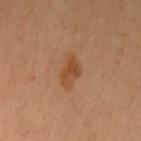workup: imaged on a skin check; not biopsied | lighting: cross-polarized | automated metrics: a lesion color around L≈48 a*≈22 b*≈37 in CIELAB, about 8 CIELAB-L* units darker than the surrounding skin, and a normalized lesion–skin contrast near 7.5; a border-irregularity index near 4/10, internal color variation of about 2.5 on a 0–10 scale, and radial color variation of about 1 | subject: female, in their 40s | imaging modality: 15 mm crop, total-body photography | size: ~3.5 mm (longest diameter) | location: the left upper arm.